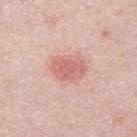A male patient, approximately 40 years of age.
Automated image analysis of the tile measured a mean CIELAB color near L≈64 a*≈26 b*≈26, roughly 10 lightness units darker than nearby skin, and a lesion-to-skin contrast of about 6 (normalized; higher = more distinct). The analysis additionally found border irregularity of about 2.5 on a 0–10 scale, a within-lesion color-variation index near 2.5/10, and radial color variation of about 1.
A roughly 15 mm field-of-view crop from a total-body skin photograph.
The lesion's longest dimension is about 4 mm.
Located on the right upper arm.